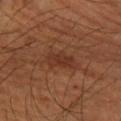The lesion was tiled from a total-body skin photograph and was not biopsied.
A 15 mm close-up tile from a total-body photography series done for melanoma screening.
From the right forearm.
A male patient, about 60 years old.
This is a cross-polarized tile.
Approximately 3.5 mm at its widest.